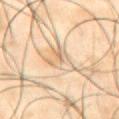  biopsy_status: not biopsied; imaged during a skin examination
  image:
    source: total-body photography crop
    field_of_view_mm: 15
  site: abdomen
  patient:
    sex: male
    age_approx: 50